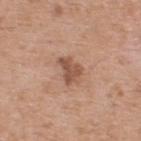The lesion was tiled from a total-body skin photograph and was not biopsied.
Captured under white-light illumination.
An algorithmic analysis of the crop reported a lesion area of about 5.5 mm², an outline eccentricity of about 0.65 (0 = round, 1 = elongated), and a shape-asymmetry score of about 0.4 (0 = symmetric).
The subject is a male in their mid- to late 50s.
The lesion's longest dimension is about 3 mm.
A close-up tile cropped from a whole-body skin photograph, about 15 mm across.
From the upper back.